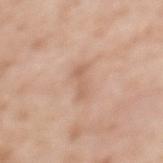This lesion was catalogued during total-body skin photography and was not selected for biopsy. The lesion is on the back. A 15 mm crop from a total-body photograph taken for skin-cancer surveillance. A female subject, approximately 40 years of age.